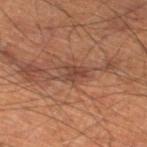| feature | finding |
|---|---|
| notes | imaged on a skin check; not biopsied |